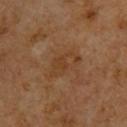Q: Is there a histopathology result?
A: imaged on a skin check; not biopsied
Q: Who is the patient?
A: male, approximately 60 years of age
Q: What is the lesion's diameter?
A: ~4.5 mm (longest diameter)
Q: What lighting was used for the tile?
A: cross-polarized
Q: What did automated image analysis measure?
A: a classifier nevus-likeness of about 0/100 and lesion-presence confidence of about 100/100
Q: Where on the body is the lesion?
A: the upper back
Q: How was this image acquired?
A: 15 mm crop, total-body photography